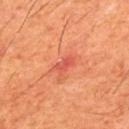This lesion was catalogued during total-body skin photography and was not selected for biopsy. This is a cross-polarized tile. A male subject about 60 years old. The lesion is located on the back. Automated tile analysis of the lesion measured an area of roughly 3.5 mm², an outline eccentricity of about 0.85 (0 = round, 1 = elongated), and a shape-asymmetry score of about 0.25 (0 = symmetric). The analysis additionally found a lesion color around L≈53 a*≈37 b*≈35 in CIELAB, roughly 8 lightness units darker than nearby skin, and a lesion-to-skin contrast of about 5.5 (normalized; higher = more distinct). And it measured a classifier nevus-likeness of about 0/100 and a detector confidence of about 100 out of 100 that the crop contains a lesion. A 15 mm close-up extracted from a 3D total-body photography capture. The recorded lesion diameter is about 2.5 mm.